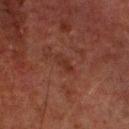Q: What kind of image is this?
A: 15 mm crop, total-body photography
Q: Lesion location?
A: the arm
Q: What did automated image analysis measure?
A: a lesion area of about 2.5 mm², an eccentricity of roughly 0.9, and a shape-asymmetry score of about 0.35 (0 = symmetric); a lesion color around L≈24 a*≈19 b*≈23 in CIELAB; a border-irregularity index near 4/10 and radial color variation of about 0; a nevus-likeness score of about 0/100
Q: How large is the lesion?
A: ≈2.5 mm
Q: What are the patient's age and sex?
A: male, approximately 80 years of age